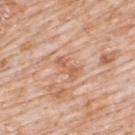This lesion was catalogued during total-body skin photography and was not selected for biopsy.
On the upper back.
The patient is a male aged approximately 80.
The lesion-visualizer software estimated a footprint of about 3.5 mm² and two-axis asymmetry of about 0.3. The analysis additionally found a mean CIELAB color near L≈61 a*≈23 b*≈34, roughly 9 lightness units darker than nearby skin, and a normalized lesion–skin contrast near 6.
The tile uses white-light illumination.
Cropped from a whole-body photographic skin survey; the tile spans about 15 mm.